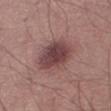Case summary:
* biopsy status · catalogued during a skin exam; not biopsied
* diameter · ~5 mm (longest diameter)
* tile lighting · white-light
* image · 15 mm crop, total-body photography
* location · the leg
* patient · male, about 55 years old